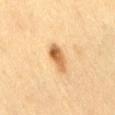Imaged during a routine full-body skin examination; the lesion was not biopsied and no histopathology is available.
A female patient aged around 40.
A 15 mm close-up extracted from a 3D total-body photography capture.
Approximately 4 mm at its widest.
Located on the lower back.